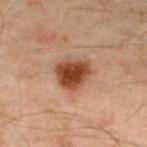Impression:
The lesion was tiled from a total-body skin photograph and was not biopsied.
Context:
Cropped from a whole-body photographic skin survey; the tile spans about 15 mm. The patient is a male aged around 45. The lesion is on the left lower leg. The total-body-photography lesion software estimated an average lesion color of about L≈39 a*≈21 b*≈29 (CIELAB), a lesion–skin lightness drop of about 14, and a normalized lesion–skin contrast near 11.5.